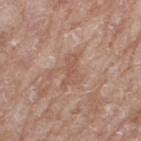  biopsy_status: not biopsied; imaged during a skin examination
  image:
    source: total-body photography crop
    field_of_view_mm: 15
  lighting: white-light
  patient:
    sex: female
    age_approx: 75
  lesion_size:
    long_diameter_mm_approx: 4.0
  site: right thigh